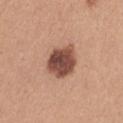follow-up — no biopsy performed (imaged during a skin exam) | subject — female, roughly 35 years of age | site — the leg | image source — ~15 mm tile from a whole-body skin photo | lighting — white-light.